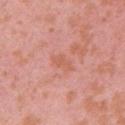Assessment:
The lesion was tiled from a total-body skin photograph and was not biopsied.
Background:
A 15 mm close-up tile from a total-body photography series done for melanoma screening. This is a white-light tile. Approximately 3 mm at its widest. The lesion is located on the chest. The total-body-photography lesion software estimated a border-irregularity index near 3/10 and a color-variation rating of about 1.5/10. A female patient, aged 38 to 42.